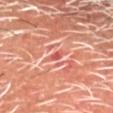The lesion was tiled from a total-body skin photograph and was not biopsied. The patient is a male aged 63 to 67. A 15 mm crop from a total-body photograph taken for skin-cancer surveillance. This is a cross-polarized tile. Located on the head or neck. Approximately 1.5 mm at its widest. Automated tile analysis of the lesion measured a footprint of about 1 mm², an eccentricity of roughly 0.85, and a shape-asymmetry score of about 0.3 (0 = symmetric). It also reported border irregularity of about 2.5 on a 0–10 scale.